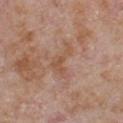Q: Is there a histopathology result?
A: total-body-photography surveillance lesion; no biopsy
Q: Lesion size?
A: about 4.5 mm
Q: Lesion location?
A: the chest
Q: What is the imaging modality?
A: ~15 mm crop, total-body skin-cancer survey
Q: What lighting was used for the tile?
A: white-light illumination
Q: What are the patient's age and sex?
A: male, about 65 years old